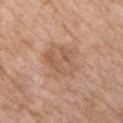The lesion was tiled from a total-body skin photograph and was not biopsied. Approximately 5 mm at its widest. The lesion-visualizer software estimated a lesion area of about 17 mm², an outline eccentricity of about 0.4 (0 = round, 1 = elongated), and a symmetry-axis asymmetry near 0.2. The software also gave an average lesion color of about L≈58 a*≈20 b*≈30 (CIELAB), about 7 CIELAB-L* units darker than the surrounding skin, and a normalized lesion–skin contrast near 5. The patient is a female aged approximately 65. Located on the chest. A 15 mm close-up extracted from a 3D total-body photography capture.